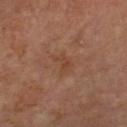Background:
Cropped from a total-body skin-imaging series; the visible field is about 15 mm. Longest diameter approximately 3 mm. The total-body-photography lesion software estimated a footprint of about 5 mm² and a symmetry-axis asymmetry near 0.4. It also reported a lesion color around L≈42 a*≈20 b*≈29 in CIELAB, a lesion–skin lightness drop of about 5, and a normalized border contrast of about 5. And it measured a border-irregularity rating of about 4.5/10 and a peripheral color-asymmetry measure near 0.5. The analysis additionally found an automated nevus-likeness rating near 0 out of 100 and a lesion-detection confidence of about 100/100. Located on the arm. The patient is a male approximately 70 years of age. The tile uses cross-polarized illumination.